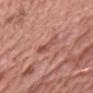Assessment: The lesion was photographed on a routine skin check and not biopsied; there is no pathology result. Background: A male subject, aged 48–52. The lesion is located on the chest. Measured at roughly 2.5 mm in maximum diameter. A 15 mm close-up extracted from a 3D total-body photography capture. The tile uses white-light illumination.